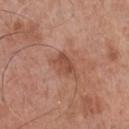Impression:
Recorded during total-body skin imaging; not selected for excision or biopsy.
Clinical summary:
A region of skin cropped from a whole-body photographic capture, roughly 15 mm wide. The patient is a male aged approximately 70. On the chest. The lesion's longest dimension is about 3 mm. The tile uses white-light illumination. Automated tile analysis of the lesion measured an area of roughly 4 mm², an eccentricity of roughly 0.8, and a symmetry-axis asymmetry near 0.3. The software also gave an average lesion color of about L≈48 a*≈24 b*≈30 (CIELAB) and a lesion-to-skin contrast of about 7 (normalized; higher = more distinct). It also reported an automated nevus-likeness rating near 5 out of 100 and a detector confidence of about 100 out of 100 that the crop contains a lesion.